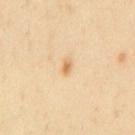Case summary:
– workup: catalogued during a skin exam; not biopsied
– acquisition: total-body-photography crop, ~15 mm field of view
– patient: male, aged approximately 45
– size: about 1.5 mm
– illumination: cross-polarized
– anatomic site: the chest
– image-analysis metrics: a footprint of about 1.5 mm² and an outline eccentricity of about 0.75 (0 = round, 1 = elongated); a mean CIELAB color near L≈69 a*≈18 b*≈42, a lesion–skin lightness drop of about 10, and a normalized lesion–skin contrast near 7.5; a border-irregularity rating of about 2/10, a color-variation rating of about 0/10, and radial color variation of about 0; an automated nevus-likeness rating near 85 out of 100 and a lesion-detection confidence of about 100/100